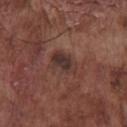The lesion was tiled from a total-body skin photograph and was not biopsied. From the chest. A male subject about 75 years old. About 3.5 mm across. Automated image analysis of the tile measured a lesion area of about 6.5 mm², an outline eccentricity of about 0.75 (0 = round, 1 = elongated), and a symmetry-axis asymmetry near 0.2. The software also gave a lesion color around L≈33 a*≈17 b*≈20 in CIELAB and roughly 8 lightness units darker than nearby skin. The analysis additionally found border irregularity of about 2.5 on a 0–10 scale and radial color variation of about 1. The software also gave a nevus-likeness score of about 20/100 and a detector confidence of about 100 out of 100 that the crop contains a lesion. Captured under white-light illumination. A close-up tile cropped from a whole-body skin photograph, about 15 mm across.